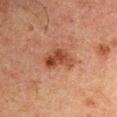Assessment: Imaged during a routine full-body skin examination; the lesion was not biopsied and no histopathology is available. Context: The tile uses cross-polarized illumination. The patient is a male aged around 50. The recorded lesion diameter is about 4 mm. The lesion is located on the arm. The lesion-visualizer software estimated a footprint of about 8.5 mm², a shape eccentricity near 0.75, and a symmetry-axis asymmetry near 0.35. It also reported a lesion color around L≈36 a*≈22 b*≈27 in CIELAB, roughly 9 lightness units darker than nearby skin, and a normalized border contrast of about 8.5. It also reported an automated nevus-likeness rating near 45 out of 100 and lesion-presence confidence of about 100/100. A lesion tile, about 15 mm wide, cut from a 3D total-body photograph.